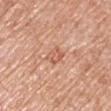Captured during whole-body skin photography for melanoma surveillance; the lesion was not biopsied. This is a white-light tile. Cropped from a total-body skin-imaging series; the visible field is about 15 mm. From the chest. Approximately 2.5 mm at its widest. A male subject approximately 70 years of age.